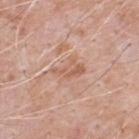<case>
<biopsy_status>not biopsied; imaged during a skin examination</biopsy_status>
<patient>
  <sex>male</sex>
  <age_approx>50</age_approx>
</patient>
<image>
  <source>total-body photography crop</source>
  <field_of_view_mm>15</field_of_view_mm>
</image>
<site>upper back</site>
</case>